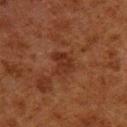Q: Was a biopsy performed?
A: no biopsy performed (imaged during a skin exam)
Q: What are the patient's age and sex?
A: male, aged around 80
Q: What is the anatomic site?
A: the left lower leg
Q: Illumination type?
A: cross-polarized
Q: What is the imaging modality?
A: 15 mm crop, total-body photography
Q: What is the lesion's diameter?
A: ~3 mm (longest diameter)